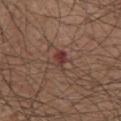The lesion was tiled from a total-body skin photograph and was not biopsied.
Located on the chest.
A lesion tile, about 15 mm wide, cut from a 3D total-body photograph.
A male patient approximately 75 years of age.